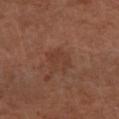Q: Is there a histopathology result?
A: catalogued during a skin exam; not biopsied
Q: Where on the body is the lesion?
A: the right lower leg
Q: How was this image acquired?
A: total-body-photography crop, ~15 mm field of view
Q: Lesion size?
A: about 3.5 mm
Q: Who is the patient?
A: male, aged 63 to 67
Q: What lighting was used for the tile?
A: cross-polarized